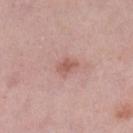Imaged during a routine full-body skin examination; the lesion was not biopsied and no histopathology is available. About 2.5 mm across. A region of skin cropped from a whole-body photographic capture, roughly 15 mm wide. A female patient, about 40 years old. An algorithmic analysis of the crop reported a footprint of about 3 mm² and an outline eccentricity of about 0.8 (0 = round, 1 = elongated). The analysis additionally found about 9 CIELAB-L* units darker than the surrounding skin and a normalized border contrast of about 6.5. And it measured a border-irregularity rating of about 3/10 and a color-variation rating of about 2/10. The software also gave an automated nevus-likeness rating near 5 out of 100. The lesion is on the leg.